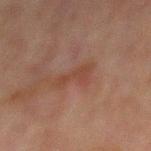This lesion was catalogued during total-body skin photography and was not selected for biopsy.
Cropped from a whole-body photographic skin survey; the tile spans about 15 mm.
A male patient, about 60 years old.
The tile uses cross-polarized illumination.
Approximately 3.5 mm at its widest.
The lesion is located on the mid back.